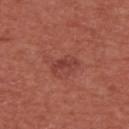Assessment:
The lesion was tiled from a total-body skin photograph and was not biopsied.
Context:
A male subject, in their mid-60s. Automated image analysis of the tile measured a footprint of about 3.5 mm² and an outline eccentricity of about 0.95 (0 = round, 1 = elongated). The software also gave an average lesion color of about L≈41 a*≈29 b*≈27 (CIELAB), about 8 CIELAB-L* units darker than the surrounding skin, and a normalized lesion–skin contrast near 6. And it measured internal color variation of about 0 on a 0–10 scale and peripheral color asymmetry of about 0. Captured under white-light illumination. From the upper back. A 15 mm crop from a total-body photograph taken for skin-cancer surveillance. Approximately 3.5 mm at its widest.